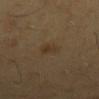Notes:
- notes — imaged on a skin check; not biopsied
- acquisition — ~15 mm crop, total-body skin-cancer survey
- patient — male, aged 63–67
- anatomic site — the mid back